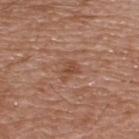The lesion was photographed on a routine skin check and not biopsied; there is no pathology result.
The lesion is located on the upper back.
About 2.5 mm across.
A male patient in their 50s.
The lesion-visualizer software estimated a footprint of about 3.5 mm², an outline eccentricity of about 0.75 (0 = round, 1 = elongated), and a symmetry-axis asymmetry near 0.45. It also reported a lesion color around L≈48 a*≈22 b*≈30 in CIELAB, a lesion–skin lightness drop of about 7, and a normalized border contrast of about 6.
A roughly 15 mm field-of-view crop from a total-body skin photograph.
This is a white-light tile.